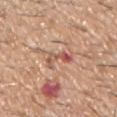Case summary:
• image: total-body-photography crop, ~15 mm field of view
• patient: male, aged approximately 60
• anatomic site: the left upper arm